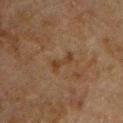biopsy status = no biopsy performed (imaged during a skin exam); body site = the chest; subject = male, approximately 50 years of age; image = ~15 mm tile from a whole-body skin photo.